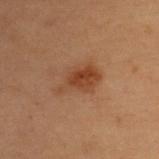notes — imaged on a skin check; not biopsied
illumination — cross-polarized
image — 15 mm crop, total-body photography
lesion size — ~5 mm (longest diameter)
body site — the upper back
subject — female, approximately 40 years of age
automated metrics — a lesion area of about 9.5 mm², an eccentricity of roughly 0.8, and two-axis asymmetry of about 0.4; a mean CIELAB color near L≈35 a*≈19 b*≈28, a lesion–skin lightness drop of about 7, and a normalized lesion–skin contrast near 7.5; a border-irregularity rating of about 4/10, internal color variation of about 3.5 on a 0–10 scale, and a peripheral color-asymmetry measure near 1.5